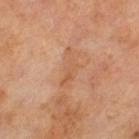This lesion was catalogued during total-body skin photography and was not selected for biopsy.
The total-body-photography lesion software estimated an area of roughly 5.5 mm² and a shape eccentricity near 0.95. The software also gave a classifier nevus-likeness of about 0/100 and a detector confidence of about 95 out of 100 that the crop contains a lesion.
Measured at roughly 4.5 mm in maximum diameter.
This is a cross-polarized tile.
This image is a 15 mm lesion crop taken from a total-body photograph.
A male patient, aged approximately 65.